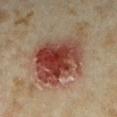The tile uses cross-polarized illumination. On the right forearm. A close-up tile cropped from a whole-body skin photograph, about 15 mm across. An algorithmic analysis of the crop reported a lesion area of about 32 mm², a shape eccentricity near 0.5, and two-axis asymmetry of about 0.25. The software also gave an average lesion color of about L≈41 a*≈24 b*≈27 (CIELAB), a lesion–skin lightness drop of about 14, and a normalized border contrast of about 11.5. It also reported a classifier nevus-likeness of about 75/100 and a detector confidence of about 100 out of 100 that the crop contains a lesion. A female patient aged around 35.